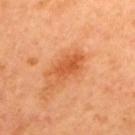Q: What is the lesion's diameter?
A: ~5 mm (longest diameter)
Q: What is the anatomic site?
A: the upper back
Q: Who is the patient?
A: male, aged approximately 65
Q: What is the imaging modality?
A: ~15 mm crop, total-body skin-cancer survey
Q: Illumination type?
A: cross-polarized